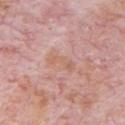Assessment:
Recorded during total-body skin imaging; not selected for excision or biopsy.
Image and clinical context:
Cropped from a total-body skin-imaging series; the visible field is about 15 mm. Automated tile analysis of the lesion measured a lesion area of about 3.5 mm², an outline eccentricity of about 0.95 (0 = round, 1 = elongated), and a shape-asymmetry score of about 0.45 (0 = symmetric). It also reported a lesion color around L≈61 a*≈22 b*≈29 in CIELAB and roughly 6 lightness units darker than nearby skin. It also reported an automated nevus-likeness rating near 0 out of 100 and lesion-presence confidence of about 100/100. The lesion is located on the front of the torso. Captured under white-light illumination. The recorded lesion diameter is about 3.5 mm. A male patient roughly 80 years of age.